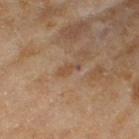Imaged during a routine full-body skin examination; the lesion was not biopsied and no histopathology is available.
Captured under cross-polarized illumination.
A female subject aged 58–62.
Cropped from a whole-body photographic skin survey; the tile spans about 15 mm.
The lesion is on the right thigh.